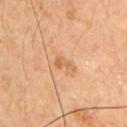| field | value |
|---|---|
| follow-up | catalogued during a skin exam; not biopsied |
| size | about 3 mm |
| subject | male, in their 60s |
| image-analysis metrics | a mean CIELAB color near L≈60 a*≈23 b*≈38 and a normalized lesion–skin contrast near 6; a border-irregularity index near 5.5/10 and a peripheral color-asymmetry measure near 0.5 |
| tile lighting | cross-polarized illumination |
| anatomic site | the right upper arm |
| imaging modality | total-body-photography crop, ~15 mm field of view |